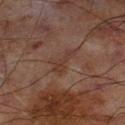The lesion was tiled from a total-body skin photograph and was not biopsied.
On the left lower leg.
A 15 mm close-up extracted from a 3D total-body photography capture.
Imaged with cross-polarized lighting.
Automated tile analysis of the lesion measured a lesion-to-skin contrast of about 5.5 (normalized; higher = more distinct). The software also gave border irregularity of about 6.5 on a 0–10 scale, a within-lesion color-variation index near 1/10, and a peripheral color-asymmetry measure near 0.5. The analysis additionally found a nevus-likeness score of about 0/100 and a detector confidence of about 90 out of 100 that the crop contains a lesion.
About 3.5 mm across.
The subject is a male aged 68–72.